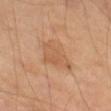Background:
The tile uses cross-polarized illumination. A male subject approximately 70 years of age. Automated image analysis of the tile measured a lesion area of about 12 mm² and two-axis asymmetry of about 0.35. It also reported a normalized border contrast of about 5. The lesion is located on the leg. A 15 mm close-up extracted from a 3D total-body photography capture.